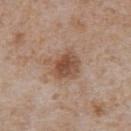Impression:
The lesion was photographed on a routine skin check and not biopsied; there is no pathology result.
Background:
A male patient, aged approximately 65. Cropped from a whole-body photographic skin survey; the tile spans about 15 mm. The lesion's longest dimension is about 4 mm. Located on the chest. An algorithmic analysis of the crop reported an area of roughly 10 mm² and an eccentricity of roughly 0.5. And it measured an average lesion color of about L≈51 a*≈19 b*≈29 (CIELAB) and a normalized lesion–skin contrast near 8. It also reported a nevus-likeness score of about 80/100 and a detector confidence of about 100 out of 100 that the crop contains a lesion. The tile uses white-light illumination.